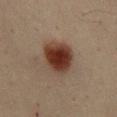notes — imaged on a skin check; not biopsied | lighting — cross-polarized illumination | size — about 5 mm | location — the upper back | subject — male, approximately 50 years of age | image — ~15 mm tile from a whole-body skin photo.